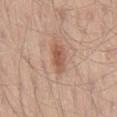Q: Was a biopsy performed?
A: catalogued during a skin exam; not biopsied
Q: How was the tile lit?
A: white-light illumination
Q: How was this image acquired?
A: ~15 mm tile from a whole-body skin photo
Q: Where on the body is the lesion?
A: the right thigh
Q: How large is the lesion?
A: ≈3.5 mm
Q: Who is the patient?
A: male, aged around 35